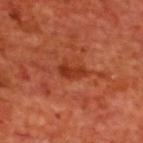{"biopsy_status": "not biopsied; imaged during a skin examination", "image": {"source": "total-body photography crop", "field_of_view_mm": 15}, "lighting": "cross-polarized", "site": "upper back", "patient": {"sex": "male", "age_approx": 70}}